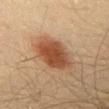notes = no biopsy performed (imaged during a skin exam)